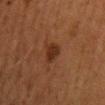{
  "biopsy_status": "not biopsied; imaged during a skin examination",
  "patient": {
    "sex": "female",
    "age_approx": 50
  },
  "lighting": "cross-polarized",
  "site": "back",
  "lesion_size": {
    "long_diameter_mm_approx": 3.0
  },
  "image": {
    "source": "total-body photography crop",
    "field_of_view_mm": 15
  },
  "automated_metrics": {
    "area_mm2_approx": 4.5,
    "eccentricity": 0.8,
    "shape_asymmetry": 0.3,
    "cielab_L": 25,
    "cielab_a": 19,
    "cielab_b": 26,
    "vs_skin_darker_L": 8.0,
    "vs_skin_contrast_norm": 8.5,
    "nevus_likeness_0_100": 85
  }
}